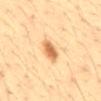biopsy status = catalogued during a skin exam; not biopsied
image source = 15 mm crop, total-body photography
location = the abdomen
illumination = cross-polarized illumination
automated lesion analysis = a footprint of about 5.5 mm², an eccentricity of roughly 0.85, and a symmetry-axis asymmetry near 0.15; an average lesion color of about L≈63 a*≈21 b*≈39 (CIELAB), about 14 CIELAB-L* units darker than the surrounding skin, and a normalized border contrast of about 8.5; a border-irregularity index near 2/10 and a color-variation rating of about 3/10
lesion size = ~3.5 mm (longest diameter)
subject = male, aged around 35